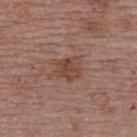Notes:
* biopsy status · imaged on a skin check; not biopsied
* image source · total-body-photography crop, ~15 mm field of view
* location · the upper back
* diameter · about 3.5 mm
* subject · female, about 65 years old
* tile lighting · white-light